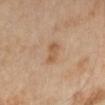Assessment: Imaged during a routine full-body skin examination; the lesion was not biopsied and no histopathology is available. Context: Located on the right lower leg. The patient is a female aged around 70. The total-body-photography lesion software estimated a footprint of about 4.5 mm², an outline eccentricity of about 0.85 (0 = round, 1 = elongated), and two-axis asymmetry of about 0.35. The software also gave border irregularity of about 3 on a 0–10 scale, internal color variation of about 2.5 on a 0–10 scale, and radial color variation of about 1. This is a cross-polarized tile. A lesion tile, about 15 mm wide, cut from a 3D total-body photograph.